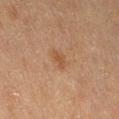Clinical summary:
This is a cross-polarized tile. A 15 mm close-up extracted from a 3D total-body photography capture. The patient is a female about 55 years old. On the abdomen.